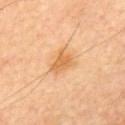The lesion was tiled from a total-body skin photograph and was not biopsied. Imaged with cross-polarized lighting. A close-up tile cropped from a whole-body skin photograph, about 15 mm across. The patient is a male aged around 60. The lesion is located on the upper back. Approximately 3.5 mm at its widest.